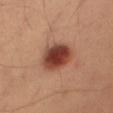A male subject, aged 38 to 42. Captured under cross-polarized illumination. About 4.5 mm across. Located on the right thigh. A close-up tile cropped from a whole-body skin photograph, about 15 mm across. An algorithmic analysis of the crop reported a lesion color around L≈42 a*≈26 b*≈29 in CIELAB, roughly 16 lightness units darker than nearby skin, and a normalized lesion–skin contrast near 12.